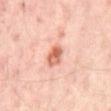notes: total-body-photography surveillance lesion; no biopsy | image: ~15 mm tile from a whole-body skin photo | patient: aged 53–57 | automated metrics: a mean CIELAB color near L≈64 a*≈28 b*≈32, roughly 14 lightness units darker than nearby skin, and a lesion-to-skin contrast of about 9 (normalized; higher = more distinct); a border-irregularity index near 2.5/10 and internal color variation of about 7.5 on a 0–10 scale; a nevus-likeness score of about 80/100 and a detector confidence of about 100 out of 100 that the crop contains a lesion | body site: the mid back | illumination: cross-polarized.